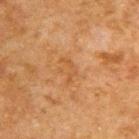The lesion was photographed on a routine skin check and not biopsied; there is no pathology result. Located on the upper back. A male subject aged around 50. Imaged with cross-polarized lighting. A 15 mm close-up extracted from a 3D total-body photography capture.